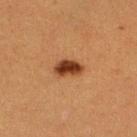Part of a total-body skin-imaging series; this lesion was reviewed on a skin check and was not flagged for biopsy.
About 3.5 mm across.
From the right lower leg.
The patient is a female aged around 40.
A 15 mm close-up tile from a total-body photography series done for melanoma screening.
Automated image analysis of the tile measured border irregularity of about 1.5 on a 0–10 scale, internal color variation of about 4 on a 0–10 scale, and a peripheral color-asymmetry measure near 1. The analysis additionally found an automated nevus-likeness rating near 100 out of 100 and lesion-presence confidence of about 100/100.
This is a cross-polarized tile.